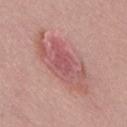Q: Was a biopsy performed?
A: catalogued during a skin exam; not biopsied
Q: What did automated image analysis measure?
A: an area of roughly 24 mm², an eccentricity of roughly 0.9, and two-axis asymmetry of about 0.25; an average lesion color of about L≈56 a*≈26 b*≈22 (CIELAB) and a normalized border contrast of about 6; border irregularity of about 5 on a 0–10 scale and internal color variation of about 5.5 on a 0–10 scale; an automated nevus-likeness rating near 20 out of 100 and a lesion-detection confidence of about 100/100
Q: How was the tile lit?
A: white-light
Q: Lesion size?
A: ≈8.5 mm
Q: What is the anatomic site?
A: the back
Q: What are the patient's age and sex?
A: male, about 40 years old
Q: How was this image acquired?
A: total-body-photography crop, ~15 mm field of view